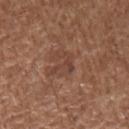Assessment: The lesion was photographed on a routine skin check and not biopsied; there is no pathology result. Image and clinical context: The lesion is on the left forearm. A female patient, aged 48–52. This is a white-light tile. A roughly 15 mm field-of-view crop from a total-body skin photograph. About 3 mm across.